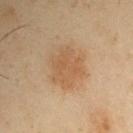biopsy status=imaged on a skin check; not biopsied | size=about 4.5 mm | tile lighting=cross-polarized illumination | site=the left upper arm | image source=total-body-photography crop, ~15 mm field of view | subject=male, aged around 55.